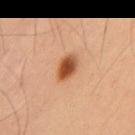Assessment:
Captured during whole-body skin photography for melanoma surveillance; the lesion was not biopsied.